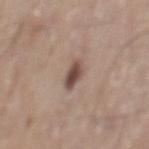Impression: Captured during whole-body skin photography for melanoma surveillance; the lesion was not biopsied. Acquisition and patient details: A close-up tile cropped from a whole-body skin photograph, about 15 mm across. The patient is a male in their mid-70s. The lesion is located on the mid back.